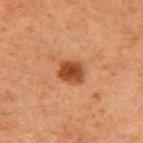Clinical impression: Recorded during total-body skin imaging; not selected for excision or biopsy. Acquisition and patient details: About 3 mm across. On the right upper arm. The tile uses cross-polarized illumination. Cropped from a total-body skin-imaging series; the visible field is about 15 mm. A male patient in their 60s.